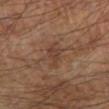{
  "biopsy_status": "not biopsied; imaged during a skin examination",
  "automated_metrics": {
    "area_mm2_approx": 4.0,
    "shape_asymmetry": 0.3,
    "cielab_L": 38,
    "cielab_a": 18,
    "cielab_b": 26,
    "vs_skin_darker_L": 6.0,
    "vs_skin_contrast_norm": 5.0
  },
  "image": {
    "source": "total-body photography crop",
    "field_of_view_mm": 15
  },
  "lesion_size": {
    "long_diameter_mm_approx": 3.5
  },
  "site": "left forearm",
  "lighting": "cross-polarized",
  "patient": {
    "sex": "male",
    "age_approx": 65
  }
}